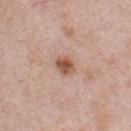The lesion was photographed on a routine skin check and not biopsied; there is no pathology result. Imaged with white-light lighting. A male subject, about 40 years old. On the chest. A roughly 15 mm field-of-view crop from a total-body skin photograph. The lesion's longest dimension is about 2.5 mm.